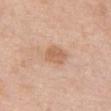Context:
Approximately 3 mm at its widest. A region of skin cropped from a whole-body photographic capture, roughly 15 mm wide. A female subject approximately 60 years of age. The lesion is on the chest.